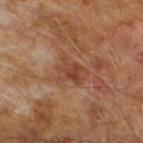Q: Is there a histopathology result?
A: catalogued during a skin exam; not biopsied
Q: What is the imaging modality?
A: 15 mm crop, total-body photography
Q: What did automated image analysis measure?
A: an area of roughly 8 mm², an eccentricity of roughly 0.65, and a symmetry-axis asymmetry near 0.45; a border-irregularity rating of about 5/10, internal color variation of about 4.5 on a 0–10 scale, and radial color variation of about 1.5
Q: Lesion size?
A: ~4 mm (longest diameter)
Q: Patient demographics?
A: male, about 65 years old
Q: Illumination type?
A: cross-polarized